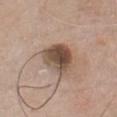follow-up: imaged on a skin check; not biopsied
site: the chest
tile lighting: white-light illumination
patient: male, in their mid- to late 70s
size: ≈4 mm
acquisition: ~15 mm tile from a whole-body skin photo